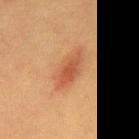No biopsy was performed on this lesion — it was imaged during a full skin examination and was not determined to be concerning. Longest diameter approximately 5.5 mm. Captured under cross-polarized illumination. A male subject aged 38 to 42. A 15 mm crop from a total-body photograph taken for skin-cancer surveillance. On the back.